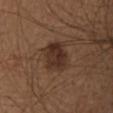This lesion was catalogued during total-body skin photography and was not selected for biopsy.
A female patient aged 38–42.
A close-up tile cropped from a whole-body skin photograph, about 15 mm across.
On the upper back.